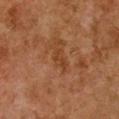No biopsy was performed on this lesion — it was imaged during a full skin examination and was not determined to be concerning. A lesion tile, about 15 mm wide, cut from a 3D total-body photograph. A female patient, in their 60s. On the front of the torso.